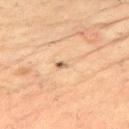workup — total-body-photography surveillance lesion; no biopsy | imaging modality — ~15 mm tile from a whole-body skin photo | patient — male, aged 48–52 | tile lighting — cross-polarized | lesion diameter — about 3 mm | site — the upper back.